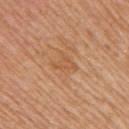This lesion was catalogued during total-body skin photography and was not selected for biopsy. A female patient roughly 55 years of age. Automated image analysis of the tile measured a lesion–skin lightness drop of about 6. The analysis additionally found a border-irregularity rating of about 4.5/10 and peripheral color asymmetry of about 0.5. From the right upper arm. The tile uses white-light illumination. A 15 mm close-up tile from a total-body photography series done for melanoma screening. Longest diameter approximately 3 mm.